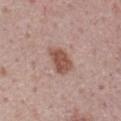Impression: The lesion was tiled from a total-body skin photograph and was not biopsied. Clinical summary: The tile uses white-light illumination. A 15 mm crop from a total-body photograph taken for skin-cancer surveillance. A male patient approximately 75 years of age. Located on the chest.